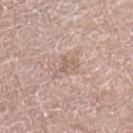follow-up: total-body-photography surveillance lesion; no biopsy
tile lighting: white-light
body site: the right lower leg
subject: female, aged 63 to 67
image source: ~15 mm tile from a whole-body skin photo
lesion diameter: ≈3.5 mm